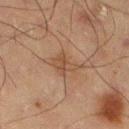biopsy status: catalogued during a skin exam; not biopsied | image source: 15 mm crop, total-body photography | image-analysis metrics: a lesion area of about 6 mm²; a lesion color around L≈39 a*≈15 b*≈26 in CIELAB and about 6 CIELAB-L* units darker than the surrounding skin; internal color variation of about 2.5 on a 0–10 scale and radial color variation of about 1; a classifier nevus-likeness of about 5/100 and a detector confidence of about 100 out of 100 that the crop contains a lesion | site: the leg | lesion diameter: ~3.5 mm (longest diameter) | patient: male, aged approximately 65 | lighting: cross-polarized.